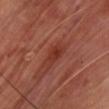{"patient": {"sex": "male", "age_approx": 70}, "image": {"source": "total-body photography crop", "field_of_view_mm": 15}, "site": "chest"}